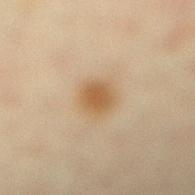Impression:
Recorded during total-body skin imaging; not selected for excision or biopsy.
Image and clinical context:
Measured at roughly 3 mm in maximum diameter. A female patient, in their mid-60s. Cropped from a total-body skin-imaging series; the visible field is about 15 mm. Located on the right lower leg. Captured under cross-polarized illumination.